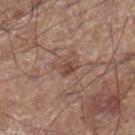biopsy status = total-body-photography surveillance lesion; no biopsy | size = ≈3 mm | imaging modality = ~15 mm crop, total-body skin-cancer survey | patient = male, about 60 years old | anatomic site = the right lower leg.